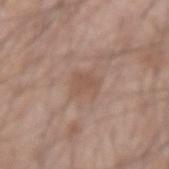automated lesion analysis = a footprint of about 6.5 mm²; a classifier nevus-likeness of about 0/100 and a detector confidence of about 100 out of 100 that the crop contains a lesion | lighting = white-light | imaging modality = ~15 mm tile from a whole-body skin photo | lesion size = ≈3 mm | anatomic site = the left forearm | subject = male, about 45 years old.